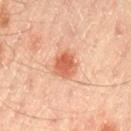Notes:
- follow-up · imaged on a skin check; not biopsied
- image-analysis metrics · a mean CIELAB color near L≈61 a*≈30 b*≈36; an automated nevus-likeness rating near 100 out of 100
- subject · male, roughly 65 years of age
- size · about 3 mm
- body site · the left thigh
- tile lighting · cross-polarized
- imaging modality · 15 mm crop, total-body photography